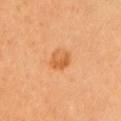* biopsy status · catalogued during a skin exam; not biopsied
* lighting · cross-polarized illumination
* subject · female, about 50 years old
* diameter · ~2.5 mm (longest diameter)
* image source · ~15 mm crop, total-body skin-cancer survey